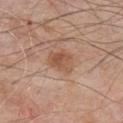Notes:
– workup · imaged on a skin check; not biopsied
– diameter · about 3.5 mm
– subject · male, aged 78–82
– image · ~15 mm crop, total-body skin-cancer survey
– site · the chest
– illumination · white-light
– TBP lesion metrics · an outline eccentricity of about 0.65 (0 = round, 1 = elongated) and a symmetry-axis asymmetry near 0.25; an average lesion color of about L≈53 a*≈21 b*≈31 (CIELAB), about 9 CIELAB-L* units darker than the surrounding skin, and a normalized lesion–skin contrast near 6.5; a border-irregularity rating of about 2.5/10, a within-lesion color-variation index near 5/10, and peripheral color asymmetry of about 2